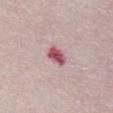Q: Is there a histopathology result?
A: total-body-photography surveillance lesion; no biopsy
Q: How large is the lesion?
A: ~3 mm (longest diameter)
Q: What did automated image analysis measure?
A: about 16 CIELAB-L* units darker than the surrounding skin and a normalized border contrast of about 10.5; a nevus-likeness score of about 0/100 and lesion-presence confidence of about 100/100
Q: What is the imaging modality?
A: ~15 mm crop, total-body skin-cancer survey
Q: Illumination type?
A: white-light
Q: Patient demographics?
A: female, in their 50s
Q: Lesion location?
A: the chest